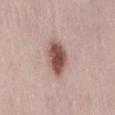follow-up: total-body-photography surveillance lesion; no biopsy
lesion diameter: ≈4.5 mm
anatomic site: the back
image: ~15 mm crop, total-body skin-cancer survey
TBP lesion metrics: an average lesion color of about L≈52 a*≈21 b*≈23 (CIELAB), a lesion–skin lightness drop of about 16, and a lesion-to-skin contrast of about 10.5 (normalized; higher = more distinct)
patient: female, aged 28 to 32